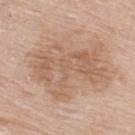Clinical impression: The lesion was photographed on a routine skin check and not biopsied; there is no pathology result. Image and clinical context: On the upper back. A roughly 15 mm field-of-view crop from a total-body skin photograph. The lesion-visualizer software estimated border irregularity of about 8.5 on a 0–10 scale and internal color variation of about 4.5 on a 0–10 scale. This is a white-light tile. A male subject aged around 80.